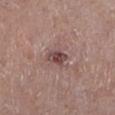notes = total-body-photography surveillance lesion; no biopsy
illumination = white-light illumination
subject = male, in their mid-60s
body site = the leg
imaging modality = total-body-photography crop, ~15 mm field of view
diameter = ~3 mm (longest diameter)